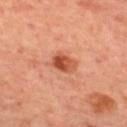* follow-up · no biopsy performed (imaged during a skin exam)
* subject · female, aged around 45
* lesion size · ~3 mm (longest diameter)
* illumination · cross-polarized
* site · the back
* image-analysis metrics · an area of roughly 5.5 mm² and an eccentricity of roughly 0.75; a mean CIELAB color near L≈51 a*≈30 b*≈36, roughly 13 lightness units darker than nearby skin, and a normalized lesion–skin contrast near 9; a classifier nevus-likeness of about 95/100 and lesion-presence confidence of about 100/100
* image source · total-body-photography crop, ~15 mm field of view